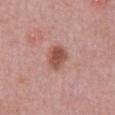Q: Is there a histopathology result?
A: no biopsy performed (imaged during a skin exam)
Q: What lighting was used for the tile?
A: white-light illumination
Q: What is the anatomic site?
A: the abdomen
Q: Who is the patient?
A: male, roughly 50 years of age
Q: What is the lesion's diameter?
A: ≈3 mm
Q: How was this image acquired?
A: ~15 mm tile from a whole-body skin photo